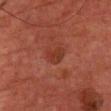Q: Is there a histopathology result?
A: imaged on a skin check; not biopsied
Q: What lighting was used for the tile?
A: cross-polarized illumination
Q: Lesion location?
A: the upper back
Q: Patient demographics?
A: male, approximately 65 years of age
Q: What kind of image is this?
A: total-body-photography crop, ~15 mm field of view
Q: Lesion size?
A: ~3 mm (longest diameter)